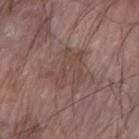Assessment: Imaged during a routine full-body skin examination; the lesion was not biopsied and no histopathology is available. Image and clinical context: Captured under white-light illumination. A roughly 15 mm field-of-view crop from a total-body skin photograph. The lesion is located on the right forearm. A male subject aged approximately 65.